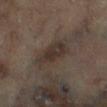Findings:
* follow-up — total-body-photography surveillance lesion; no biopsy
* diameter — ~5.5 mm (longest diameter)
* image source — ~15 mm tile from a whole-body skin photo
* body site — the right lower leg
* automated lesion analysis — a lesion area of about 9 mm², an eccentricity of roughly 0.9, and a shape-asymmetry score of about 0.3 (0 = symmetric); a classifier nevus-likeness of about 5/100 and a detector confidence of about 80 out of 100 that the crop contains a lesion
* patient — male, in their mid- to late 60s